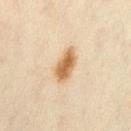<tbp_lesion>
<biopsy_status>not biopsied; imaged during a skin examination</biopsy_status>
<site>mid back</site>
<lesion_size>
  <long_diameter_mm_approx>4.0</long_diameter_mm_approx>
</lesion_size>
<automated_metrics>
  <area_mm2_approx>7.0</area_mm2_approx>
  <eccentricity>0.85</eccentricity>
  <shape_asymmetry>0.25</shape_asymmetry>
  <vs_skin_contrast_norm>10.0</vs_skin_contrast_norm>
  <color_variation_0_10>3.5</color_variation_0_10>
  <peripheral_color_asymmetry>1.0</peripheral_color_asymmetry>
  <nevus_likeness_0_100>100</nevus_likeness_0_100>
  <lesion_detection_confidence_0_100>100</lesion_detection_confidence_0_100>
</automated_metrics>
<lighting>cross-polarized</lighting>
<image>
  <source>total-body photography crop</source>
  <field_of_view_mm>15</field_of_view_mm>
</image>
<patient>
  <sex>female</sex>
  <age_approx>35</age_approx>
</patient>
</tbp_lesion>